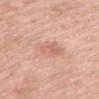The lesion was photographed on a routine skin check and not biopsied; there is no pathology result.